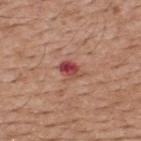The lesion was tiled from a total-body skin photograph and was not biopsied. Cropped from a whole-body photographic skin survey; the tile spans about 15 mm. An algorithmic analysis of the crop reported roughly 13 lightness units darker than nearby skin and a normalized lesion–skin contrast near 9.5. And it measured a nevus-likeness score of about 0/100 and a lesion-detection confidence of about 100/100. The lesion's longest dimension is about 2.5 mm. The tile uses white-light illumination. A male subject, in their 50s. Located on the upper back.